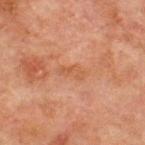Captured during whole-body skin photography for melanoma surveillance; the lesion was not biopsied. A male patient in their mid-60s. Cropped from a whole-body photographic skin survey; the tile spans about 15 mm. Located on the chest.